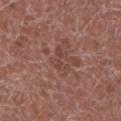Q: Illumination type?
A: white-light illumination
Q: Lesion location?
A: the left lower leg
Q: What are the patient's age and sex?
A: male, about 75 years old
Q: What kind of image is this?
A: ~15 mm crop, total-body skin-cancer survey
Q: Lesion size?
A: about 3 mm